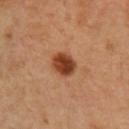Captured during whole-body skin photography for melanoma surveillance; the lesion was not biopsied. The recorded lesion diameter is about 3 mm. This image is a 15 mm lesion crop taken from a total-body photograph. Located on the chest. A male subject aged 48–52. The total-body-photography lesion software estimated a lesion area of about 7 mm², a shape eccentricity near 0.6, and a symmetry-axis asymmetry near 0.1.